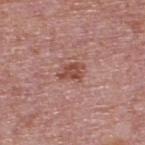{
  "biopsy_status": "not biopsied; imaged during a skin examination",
  "patient": {
    "sex": "male",
    "age_approx": 75
  },
  "lesion_size": {
    "long_diameter_mm_approx": 3.0
  },
  "automated_metrics": {
    "area_mm2_approx": 4.5,
    "eccentricity": 0.65,
    "shape_asymmetry": 0.3,
    "cielab_L": 49,
    "cielab_a": 25,
    "cielab_b": 26,
    "vs_skin_darker_L": 10.0,
    "vs_skin_contrast_norm": 8.0,
    "nevus_likeness_0_100": 50
  },
  "site": "upper back",
  "image": {
    "source": "total-body photography crop",
    "field_of_view_mm": 15
  }
}